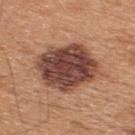{
  "biopsy_status": "not biopsied; imaged during a skin examination",
  "site": "upper back",
  "image": {
    "source": "total-body photography crop",
    "field_of_view_mm": 15
  },
  "patient": {
    "sex": "male",
    "age_approx": 45
  },
  "lesion_size": {
    "long_diameter_mm_approx": 6.5
  },
  "automated_metrics": {
    "border_irregularity_0_10": 2.0,
    "color_variation_0_10": 6.0,
    "peripheral_color_asymmetry": 2.0,
    "nevus_likeness_0_100": 25,
    "lesion_detection_confidence_0_100": 100
  }
}